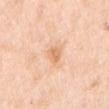notes: no biopsy performed (imaged during a skin exam); lighting: white-light illumination; subject: female, about 50 years old; anatomic site: the right upper arm; image: total-body-photography crop, ~15 mm field of view.